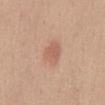Recorded during total-body skin imaging; not selected for excision or biopsy.
Captured under white-light illumination.
A close-up tile cropped from a whole-body skin photograph, about 15 mm across.
A female patient, aged 48 to 52.
The lesion is located on the front of the torso.